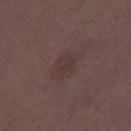Imaged during a routine full-body skin examination; the lesion was not biopsied and no histopathology is available. Located on the leg. A female subject, aged 28–32. The lesion's longest dimension is about 3 mm. The total-body-photography lesion software estimated a footprint of about 5 mm². The analysis additionally found an average lesion color of about L≈34 a*≈16 b*≈18 (CIELAB), roughly 5 lightness units darker than nearby skin, and a normalized lesion–skin contrast near 5. Captured under white-light illumination. A close-up tile cropped from a whole-body skin photograph, about 15 mm across.